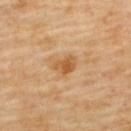A region of skin cropped from a whole-body photographic capture, roughly 15 mm wide. The lesion is located on the upper back. A female patient, aged around 60. Measured at roughly 2.5 mm in maximum diameter. Imaged with cross-polarized lighting.